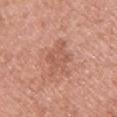biopsy status = no biopsy performed (imaged during a skin exam)
imaging modality = ~15 mm tile from a whole-body skin photo
subject = female, aged approximately 40
image-analysis metrics = a lesion-detection confidence of about 100/100
anatomic site = the front of the torso